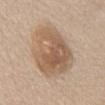Imaged during a routine full-body skin examination; the lesion was not biopsied and no histopathology is available.